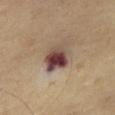<case>
<biopsy_status>not biopsied; imaged during a skin examination</biopsy_status>
</case>